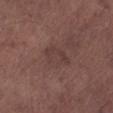Findings:
* notes — no biopsy performed (imaged during a skin exam)
* location — the right lower leg
* image source — ~15 mm crop, total-body skin-cancer survey
* illumination — white-light illumination
* TBP lesion metrics — an average lesion color of about L≈38 a*≈18 b*≈20 (CIELAB)
* size — ≈4 mm
* subject — male, in their mid- to late 50s